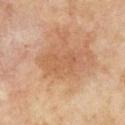Image and clinical context:
The subject is a male roughly 70 years of age. This is a cross-polarized tile. Automated tile analysis of the lesion measured an average lesion color of about L≈57 a*≈21 b*≈35 (CIELAB), a lesion–skin lightness drop of about 6, and a normalized lesion–skin contrast near 4.5. And it measured a border-irregularity index near 4.5/10, internal color variation of about 3 on a 0–10 scale, and radial color variation of about 1. Located on the chest. A lesion tile, about 15 mm wide, cut from a 3D total-body photograph. Approximately 5 mm at its widest.